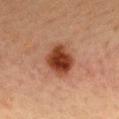Findings:
- notes · total-body-photography surveillance lesion; no biopsy
- lesion diameter · ≈4 mm
- image-analysis metrics · a footprint of about 12 mm² and a symmetry-axis asymmetry near 0.15; an average lesion color of about L≈35 a*≈23 b*≈29 (CIELAB) and roughly 14 lightness units darker than nearby skin; a border-irregularity rating of about 1.5/10, internal color variation of about 5 on a 0–10 scale, and radial color variation of about 1.5
- imaging modality · ~15 mm crop, total-body skin-cancer survey
- site · the chest
- subject · female, aged 38 to 42
- lighting · cross-polarized illumination